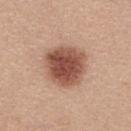This lesion was catalogued during total-body skin photography and was not selected for biopsy. From the upper back. Imaged with white-light lighting. A female subject, in their mid- to late 30s. A lesion tile, about 15 mm wide, cut from a 3D total-body photograph. The lesion-visualizer software estimated a mean CIELAB color near L≈51 a*≈23 b*≈28, roughly 16 lightness units darker than nearby skin, and a normalized border contrast of about 11.